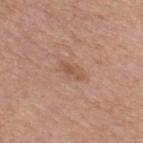Clinical impression: Recorded during total-body skin imaging; not selected for excision or biopsy. Background: Longest diameter approximately 3 mm. The patient is a male roughly 50 years of age. The tile uses white-light illumination. A 15 mm close-up tile from a total-body photography series done for melanoma screening. On the back.